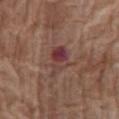workup: catalogued during a skin exam; not biopsied
patient: female, in their mid-70s
lighting: white-light illumination
lesion size: ~4 mm (longest diameter)
image: total-body-photography crop, ~15 mm field of view
TBP lesion metrics: a border-irregularity index near 4/10 and internal color variation of about 8.5 on a 0–10 scale; a lesion-detection confidence of about 100/100
site: the abdomen